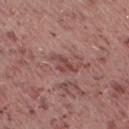No biopsy was performed on this lesion — it was imaged during a full skin examination and was not determined to be concerning. The lesion is located on the right thigh. This is a white-light tile. A region of skin cropped from a whole-body photographic capture, roughly 15 mm wide. A female patient, about 20 years old.